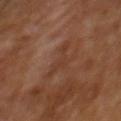Assessment: This lesion was catalogued during total-body skin photography and was not selected for biopsy. Background: The tile uses cross-polarized illumination. A lesion tile, about 15 mm wide, cut from a 3D total-body photograph. A male subject aged 63–67. An algorithmic analysis of the crop reported a border-irregularity rating of about 9/10, a within-lesion color-variation index near 0.5/10, and peripheral color asymmetry of about 0. The analysis additionally found an automated nevus-likeness rating near 0 out of 100 and a lesion-detection confidence of about 65/100. The lesion is located on the back.